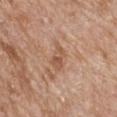Q: Was this lesion biopsied?
A: total-body-photography surveillance lesion; no biopsy
Q: Lesion size?
A: ≈3 mm
Q: Automated lesion metrics?
A: border irregularity of about 3 on a 0–10 scale, a color-variation rating of about 4/10, and radial color variation of about 1.5; a nevus-likeness score of about 0/100 and a lesion-detection confidence of about 100/100
Q: What are the patient's age and sex?
A: female, roughly 85 years of age or older
Q: What is the imaging modality?
A: total-body-photography crop, ~15 mm field of view
Q: What is the anatomic site?
A: the upper back